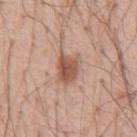Clinical impression: Captured during whole-body skin photography for melanoma surveillance; the lesion was not biopsied. Acquisition and patient details: Imaged with white-light lighting. A 15 mm crop from a total-body photograph taken for skin-cancer surveillance. The lesion is located on the chest. Measured at roughly 4 mm in maximum diameter. A male subject, about 55 years old. The total-body-photography lesion software estimated a lesion area of about 9 mm² and a symmetry-axis asymmetry near 0.25. It also reported about 12 CIELAB-L* units darker than the surrounding skin and a normalized border contrast of about 8. It also reported a border-irregularity rating of about 3/10, internal color variation of about 4.5 on a 0–10 scale, and peripheral color asymmetry of about 1.5. It also reported an automated nevus-likeness rating near 95 out of 100 and a detector confidence of about 100 out of 100 that the crop contains a lesion.